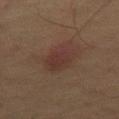{
  "biopsy_status": "not biopsied; imaged during a skin examination",
  "lighting": "cross-polarized",
  "image": {
    "source": "total-body photography crop",
    "field_of_view_mm": 15
  },
  "site": "right thigh",
  "patient": {
    "sex": "female",
    "age_approx": 55
  },
  "lesion_size": {
    "long_diameter_mm_approx": 4.5
  }
}